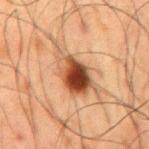biopsy status = imaged on a skin check; not biopsied
anatomic site = the mid back
subject = male, aged 58 to 62
imaging modality = ~15 mm crop, total-body skin-cancer survey
diameter = ~7 mm (longest diameter)